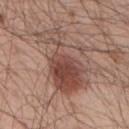{"biopsy_status": "not biopsied; imaged during a skin examination", "image": {"source": "total-body photography crop", "field_of_view_mm": 15}, "patient": {"sex": "male", "age_approx": 55}, "site": "back", "lighting": "white-light", "lesion_size": {"long_diameter_mm_approx": 8.5}, "automated_metrics": {"eccentricity": 0.85, "shape_asymmetry": 0.55, "cielab_L": 47, "cielab_a": 20, "cielab_b": 25, "vs_skin_darker_L": 11.0, "vs_skin_contrast_norm": 8.5, "nevus_likeness_0_100": 100}}